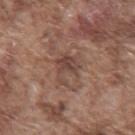The lesion was tiled from a total-body skin photograph and was not biopsied. A male subject, approximately 75 years of age. The lesion's longest dimension is about 4 mm. An algorithmic analysis of the crop reported a border-irregularity index near 7.5/10, internal color variation of about 3.5 on a 0–10 scale, and peripheral color asymmetry of about 1.5. It also reported an automated nevus-likeness rating near 0 out of 100 and a lesion-detection confidence of about 100/100. A 15 mm close-up tile from a total-body photography series done for melanoma screening. From the chest. The tile uses white-light illumination.